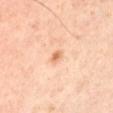The lesion was tiled from a total-body skin photograph and was not biopsied. The recorded lesion diameter is about 2 mm. A male patient, in their mid-40s. Cropped from a whole-body photographic skin survey; the tile spans about 15 mm. The lesion is located on the left upper arm. This is a cross-polarized tile.